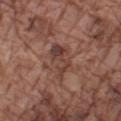Assessment: Imaged during a routine full-body skin examination; the lesion was not biopsied and no histopathology is available. Background: From the arm. A male patient aged 73 to 77. A close-up tile cropped from a whole-body skin photograph, about 15 mm across.